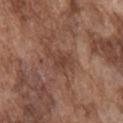Findings:
• follow-up — imaged on a skin check; not biopsied
• tile lighting — white-light
• imaging modality — total-body-photography crop, ~15 mm field of view
• subject — male, aged 73–77
• TBP lesion metrics — an average lesion color of about L≈42 a*≈20 b*≈26 (CIELAB), about 6 CIELAB-L* units darker than the surrounding skin, and a normalized border contrast of about 5.5; border irregularity of about 5.5 on a 0–10 scale, a color-variation rating of about 2/10, and radial color variation of about 0.5
• body site — the chest
• lesion diameter — about 3.5 mm